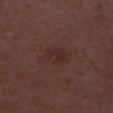The lesion was tiled from a total-body skin photograph and was not biopsied.
A roughly 15 mm field-of-view crop from a total-body skin photograph.
The patient is a male about 50 years old.
Longest diameter approximately 4 mm.